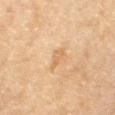Clinical impression: The lesion was tiled from a total-body skin photograph and was not biopsied. Image and clinical context: On the arm. A female patient, aged around 80. Captured under cross-polarized illumination. Measured at roughly 2.5 mm in maximum diameter. A 15 mm close-up extracted from a 3D total-body photography capture.